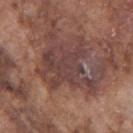{
  "biopsy_status": "not biopsied; imaged during a skin examination",
  "lesion_size": {
    "long_diameter_mm_approx": 6.5
  },
  "automated_metrics": {
    "area_mm2_approx": 21.0,
    "eccentricity": 0.55,
    "shape_asymmetry": 0.45,
    "cielab_L": 40,
    "cielab_a": 20,
    "cielab_b": 20,
    "vs_skin_darker_L": 8.0,
    "vs_skin_contrast_norm": 7.5,
    "nevus_likeness_0_100": 0,
    "lesion_detection_confidence_0_100": 70
  },
  "lighting": "white-light",
  "patient": {
    "sex": "male",
    "age_approx": 75
  },
  "image": {
    "source": "total-body photography crop",
    "field_of_view_mm": 15
  },
  "site": "right upper arm"
}